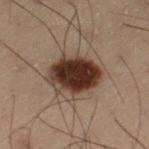Findings:
• image source · ~15 mm crop, total-body skin-cancer survey
• patient · male, roughly 55 years of age
• lesion size · about 6 mm
• body site · the left thigh